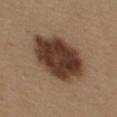Part of a total-body skin-imaging series; this lesion was reviewed on a skin check and was not flagged for biopsy. Cropped from a total-body skin-imaging series; the visible field is about 15 mm. The total-body-photography lesion software estimated an area of roughly 28 mm², an eccentricity of roughly 0.75, and a shape-asymmetry score of about 0.1 (0 = symmetric). The software also gave a border-irregularity index near 1.5/10 and internal color variation of about 5 on a 0–10 scale. The analysis additionally found a nevus-likeness score of about 65/100 and lesion-presence confidence of about 100/100. Captured under white-light illumination. On the upper back. A female patient roughly 40 years of age.